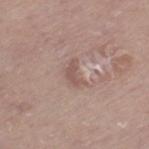Captured during whole-body skin photography for melanoma surveillance; the lesion was not biopsied.
A male subject aged approximately 75.
From the left thigh.
A roughly 15 mm field-of-view crop from a total-body skin photograph.